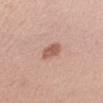The lesion was tiled from a total-body skin photograph and was not biopsied.
The lesion's longest dimension is about 3 mm.
A 15 mm close-up extracted from a 3D total-body photography capture.
The subject is a female aged 28 to 32.
This is a white-light tile.
Located on the arm.